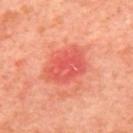Imaged during a routine full-body skin examination; the lesion was not biopsied and no histopathology is available. From the mid back. A region of skin cropped from a whole-body photographic capture, roughly 15 mm wide. A male subject, approximately 65 years of age.